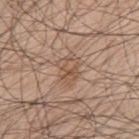Findings:
– workup — imaged on a skin check; not biopsied
– patient — male, aged approximately 70
– illumination — white-light
– site — the mid back
– size — about 3.5 mm
– image source — ~15 mm tile from a whole-body skin photo
– TBP lesion metrics — an eccentricity of roughly 0.7 and two-axis asymmetry of about 0.3; a border-irregularity rating of about 3/10, a color-variation rating of about 5.5/10, and a peripheral color-asymmetry measure near 2; an automated nevus-likeness rating near 0 out of 100 and lesion-presence confidence of about 95/100